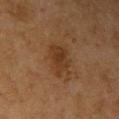Acquisition and patient details:
Approximately 4.5 mm at its widest. On the right upper arm. This is a cross-polarized tile. A 15 mm close-up tile from a total-body photography series done for melanoma screening. The total-body-photography lesion software estimated an outline eccentricity of about 0.75 (0 = round, 1 = elongated) and a symmetry-axis asymmetry near 0.25. The software also gave a lesion color around L≈32 a*≈17 b*≈29 in CIELAB, about 7 CIELAB-L* units darker than the surrounding skin, and a lesion-to-skin contrast of about 7 (normalized; higher = more distinct). The software also gave border irregularity of about 3.5 on a 0–10 scale, internal color variation of about 3 on a 0–10 scale, and peripheral color asymmetry of about 1. It also reported a nevus-likeness score of about 15/100. A female subject in their 60s.